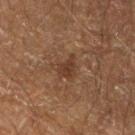| feature | finding |
|---|---|
| workup | total-body-photography surveillance lesion; no biopsy |
| lesion size | ~3 mm (longest diameter) |
| site | the left lower leg |
| image source | ~15 mm tile from a whole-body skin photo |
| automated lesion analysis | a footprint of about 4 mm², a shape eccentricity near 0.75, and two-axis asymmetry of about 0.45; border irregularity of about 4 on a 0–10 scale, a color-variation rating of about 2/10, and radial color variation of about 1; a detector confidence of about 100 out of 100 that the crop contains a lesion |
| subject | male, in their mid- to late 60s |